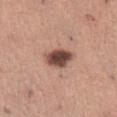Assessment:
Part of a total-body skin-imaging series; this lesion was reviewed on a skin check and was not flagged for biopsy.
Background:
Approximately 4 mm at its widest. A 15 mm close-up extracted from a 3D total-body photography capture. Located on the abdomen. An algorithmic analysis of the crop reported a footprint of about 8 mm², an outline eccentricity of about 0.75 (0 = round, 1 = elongated), and a shape-asymmetry score of about 0.2 (0 = symmetric). The tile uses white-light illumination. The subject is a female aged around 30.